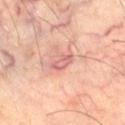Located on the right thigh. Captured under cross-polarized illumination. A male patient aged 58 to 62. An algorithmic analysis of the crop reported a footprint of about 3 mm² and an outline eccentricity of about 0.95 (0 = round, 1 = elongated). The analysis additionally found a lesion–skin lightness drop of about 9. The analysis additionally found border irregularity of about 4 on a 0–10 scale and internal color variation of about 0 on a 0–10 scale. Cropped from a total-body skin-imaging series; the visible field is about 15 mm.